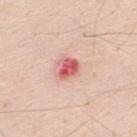workup = imaged on a skin check; not biopsied.